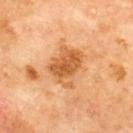Q: Was this lesion biopsied?
A: catalogued during a skin exam; not biopsied
Q: Patient demographics?
A: male, approximately 70 years of age
Q: How was the tile lit?
A: cross-polarized illumination
Q: What did automated image analysis measure?
A: a lesion area of about 13 mm², an eccentricity of roughly 0.65, and two-axis asymmetry of about 0.3; a within-lesion color-variation index near 5.5/10 and peripheral color asymmetry of about 2
Q: What is the anatomic site?
A: the upper back
Q: What is the lesion's diameter?
A: ~4.5 mm (longest diameter)
Q: How was this image acquired?
A: ~15 mm tile from a whole-body skin photo